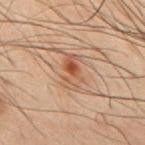Findings:
* image — ~15 mm tile from a whole-body skin photo
* subject — male, aged around 50
* site — the upper back